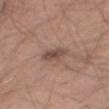Q: Was a biopsy performed?
A: imaged on a skin check; not biopsied